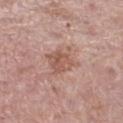Recorded during total-body skin imaging; not selected for excision or biopsy. This is a white-light tile. A roughly 15 mm field-of-view crop from a total-body skin photograph. Automated tile analysis of the lesion measured lesion-presence confidence of about 100/100. From the left thigh. A female subject in their 60s. Measured at roughly 4 mm in maximum diameter.